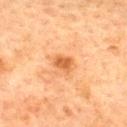Recorded during total-body skin imaging; not selected for excision or biopsy. The lesion is on the back. Longest diameter approximately 2.5 mm. A female patient, aged 58–62. Captured under cross-polarized illumination. A 15 mm close-up tile from a total-body photography series done for melanoma screening. An algorithmic analysis of the crop reported a lesion area of about 4.5 mm², a shape eccentricity near 0.6, and two-axis asymmetry of about 0.2. It also reported an average lesion color of about L≈51 a*≈24 b*≈38 (CIELAB) and a normalized border contrast of about 7.5.